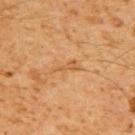Q: Was a biopsy performed?
A: total-body-photography surveillance lesion; no biopsy
Q: What is the anatomic site?
A: the back
Q: How was this image acquired?
A: 15 mm crop, total-body photography
Q: How large is the lesion?
A: ~3 mm (longest diameter)
Q: How was the tile lit?
A: cross-polarized illumination
Q: What are the patient's age and sex?
A: male, in their 60s